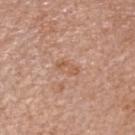Impression: This lesion was catalogued during total-body skin photography and was not selected for biopsy. Image and clinical context: The subject is a female approximately 75 years of age. On the head or neck. A 15 mm close-up extracted from a 3D total-body photography capture. The lesion's longest dimension is about 3 mm. Captured under white-light illumination. The total-body-photography lesion software estimated an eccentricity of roughly 0.9 and a symmetry-axis asymmetry near 0.4. The analysis additionally found a classifier nevus-likeness of about 0/100 and a detector confidence of about 100 out of 100 that the crop contains a lesion.